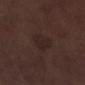Captured during whole-body skin photography for melanoma surveillance; the lesion was not biopsied. The lesion is located on the right lower leg. A 15 mm close-up extracted from a 3D total-body photography capture. The recorded lesion diameter is about 3.5 mm. Imaged with white-light lighting. A male patient in their 70s.